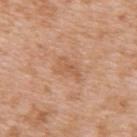– follow-up · imaged on a skin check; not biopsied
– anatomic site · the upper back
– diameter · ~3 mm (longest diameter)
– patient · female, aged approximately 40
– lighting · white-light illumination
– image · ~15 mm tile from a whole-body skin photo